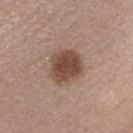Findings:
• biopsy status · imaged on a skin check; not biopsied
• TBP lesion metrics · an average lesion color of about L≈46 a*≈18 b*≈27 (CIELAB), roughly 14 lightness units darker than nearby skin, and a normalized border contrast of about 10; a border-irregularity index near 2/10, a color-variation rating of about 3.5/10, and radial color variation of about 1
• patient · male, aged around 40
• size · ~4 mm (longest diameter)
• lighting · white-light illumination
• imaging modality · total-body-photography crop, ~15 mm field of view
• location · the front of the torso